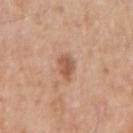Clinical impression:
Captured during whole-body skin photography for melanoma surveillance; the lesion was not biopsied.
Context:
A male patient, aged 73 to 77. On the right upper arm. An algorithmic analysis of the crop reported an outline eccentricity of about 0.5 (0 = round, 1 = elongated) and a symmetry-axis asymmetry near 0.2. The software also gave a lesion color around L≈56 a*≈22 b*≈32 in CIELAB and a lesion-to-skin contrast of about 7.5 (normalized; higher = more distinct). The analysis additionally found a nevus-likeness score of about 45/100 and a lesion-detection confidence of about 100/100. A roughly 15 mm field-of-view crop from a total-body skin photograph. The tile uses white-light illumination. Measured at roughly 2.5 mm in maximum diameter.